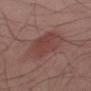Findings:
* imaging modality · 15 mm crop, total-body photography
* patient · male, in their 50s
* location · the arm